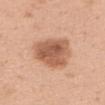notes: total-body-photography surveillance lesion; no biopsy
illumination: white-light illumination
subject: female, aged 48 to 52
image: 15 mm crop, total-body photography
automated metrics: a footprint of about 17 mm²; a mean CIELAB color near L≈58 a*≈23 b*≈33 and a lesion-to-skin contrast of about 8.5 (normalized; higher = more distinct); a within-lesion color-variation index near 4.5/10 and a peripheral color-asymmetry measure near 1.5
diameter: ~6 mm (longest diameter)
body site: the upper back